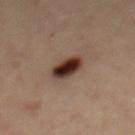{"biopsy_status": "not biopsied; imaged during a skin examination", "lighting": "cross-polarized", "site": "mid back", "patient": {"sex": "female", "age_approx": 45}, "image": {"source": "total-body photography crop", "field_of_view_mm": 15}}